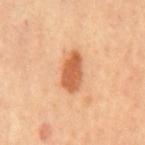Imaged during a routine full-body skin examination; the lesion was not biopsied and no histopathology is available.
The lesion is on the mid back.
The subject is a male approximately 70 years of age.
This image is a 15 mm lesion crop taken from a total-body photograph.